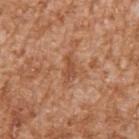- notes — catalogued during a skin exam; not biopsied
- image source — ~15 mm crop, total-body skin-cancer survey
- lesion size — ≈3 mm
- body site — the right upper arm
- patient — male, aged around 45
- illumination — white-light illumination